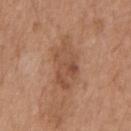Assessment:
Imaged during a routine full-body skin examination; the lesion was not biopsied and no histopathology is available.
Context:
Located on the chest. Captured under white-light illumination. A 15 mm close-up extracted from a 3D total-body photography capture. Approximately 6 mm at its widest. A male patient aged 68 to 72.